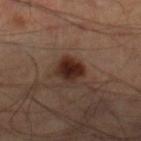<case>
  <biopsy_status>not biopsied; imaged during a skin examination</biopsy_status>
  <image>
    <source>total-body photography crop</source>
    <field_of_view_mm>15</field_of_view_mm>
  </image>
  <automated_metrics>
    <cielab_L>26</cielab_L>
    <cielab_a>19</cielab_a>
    <cielab_b>23</cielab_b>
    <vs_skin_darker_L>13.0</vs_skin_darker_L>
    <vs_skin_contrast_norm>13.0</vs_skin_contrast_norm>
    <nevus_likeness_0_100>95</nevus_likeness_0_100>
  </automated_metrics>
  <site>left leg</site>
  <patient>
    <sex>male</sex>
    <age_approx>50</age_approx>
  </patient>
  <lighting>cross-polarized</lighting>
</case>